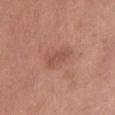biopsy_status: not biopsied; imaged during a skin examination
lesion_size:
  long_diameter_mm_approx: 2.5
image:
  source: total-body photography crop
  field_of_view_mm: 15
site: right thigh
patient:
  sex: female
  age_approx: 50
lighting: white-light